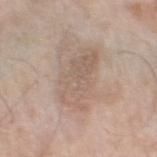Captured during whole-body skin photography for melanoma surveillance; the lesion was not biopsied. About 6 mm across. Located on the left thigh. A male patient aged approximately 70. The lesion-visualizer software estimated an area of roughly 14 mm², a shape eccentricity near 0.9, and two-axis asymmetry of about 0.3. It also reported a lesion color around L≈59 a*≈15 b*≈25 in CIELAB, a lesion–skin lightness drop of about 8, and a normalized border contrast of about 5. The analysis additionally found a within-lesion color-variation index near 3.5/10 and radial color variation of about 1.5. And it measured an automated nevus-likeness rating near 0 out of 100 and a lesion-detection confidence of about 90/100. A 15 mm crop from a total-body photograph taken for skin-cancer surveillance. This is a white-light tile.